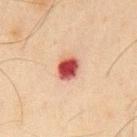notes: catalogued during a skin exam; not biopsied | illumination: cross-polarized illumination | lesion size: about 3 mm | body site: the chest | image source: ~15 mm crop, total-body skin-cancer survey | patient: male, approximately 65 years of age.